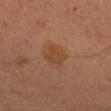Q: Was a biopsy performed?
A: catalogued during a skin exam; not biopsied
Q: What are the patient's age and sex?
A: female, aged around 55
Q: What lighting was used for the tile?
A: cross-polarized
Q: How large is the lesion?
A: about 2.5 mm
Q: What kind of image is this?
A: ~15 mm tile from a whole-body skin photo
Q: Lesion location?
A: the left upper arm